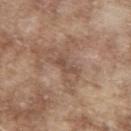biopsy status — imaged on a skin check; not biopsied | body site — the upper back | lesion size — ~3 mm (longest diameter) | subject — male, approximately 65 years of age | illumination — white-light | image source — ~15 mm tile from a whole-body skin photo | automated lesion analysis — a lesion–skin lightness drop of about 8; border irregularity of about 6.5 on a 0–10 scale, a color-variation rating of about 0/10, and radial color variation of about 0.